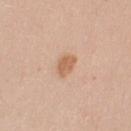Case summary:
- follow-up: no biopsy performed (imaged during a skin exam)
- subject: female, in their 40s
- automated lesion analysis: a lesion area of about 4.5 mm² and an outline eccentricity of about 0.65 (0 = round, 1 = elongated)
- anatomic site: the left upper arm
- size: about 3 mm
- image: 15 mm crop, total-body photography
- lighting: white-light